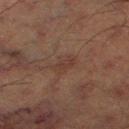Clinical impression:
Part of a total-body skin-imaging series; this lesion was reviewed on a skin check and was not flagged for biopsy.
Context:
Approximately 3 mm at its widest. On the left thigh. A male patient aged 63–67. The tile uses cross-polarized illumination. Automated image analysis of the tile measured a shape eccentricity near 0.85 and two-axis asymmetry of about 0.2. The software also gave a border-irregularity rating of about 3/10 and a color-variation rating of about 2/10. It also reported a nevus-likeness score of about 0/100. A region of skin cropped from a whole-body photographic capture, roughly 15 mm wide.